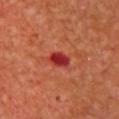Q: Is there a histopathology result?
A: imaged on a skin check; not biopsied
Q: How was this image acquired?
A: ~15 mm tile from a whole-body skin photo
Q: Lesion size?
A: ≈3 mm
Q: Lesion location?
A: the front of the torso
Q: Who is the patient?
A: female, in their mid-50s
Q: How was the tile lit?
A: cross-polarized illumination